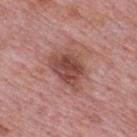biopsy status=total-body-photography surveillance lesion; no biopsy | body site=the back | patient=male, aged around 75 | lesion diameter=≈5.5 mm | tile lighting=white-light illumination | image source=15 mm crop, total-body photography.